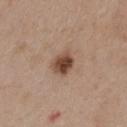notes = catalogued during a skin exam; not biopsied | lesion size = ≈3 mm | illumination = white-light | TBP lesion metrics = a lesion area of about 6 mm², an eccentricity of roughly 0.5, and two-axis asymmetry of about 0.2 | subject = female, in their mid- to late 40s | site = the back | acquisition = ~15 mm tile from a whole-body skin photo.